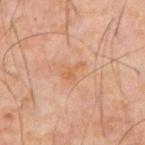Recorded during total-body skin imaging; not selected for excision or biopsy.
A 15 mm close-up extracted from a 3D total-body photography capture.
A male patient, aged approximately 60.
Automated tile analysis of the lesion measured a footprint of about 3 mm², a shape eccentricity near 0.85, and a shape-asymmetry score of about 0.55 (0 = symmetric). And it measured border irregularity of about 6 on a 0–10 scale and a peripheral color-asymmetry measure near 0.5. The analysis additionally found an automated nevus-likeness rating near 0 out of 100.
Located on the abdomen.
About 2.5 mm across.